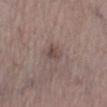Impression:
Imaged during a routine full-body skin examination; the lesion was not biopsied and no histopathology is available.
Background:
The lesion-visualizer software estimated a lesion color around L≈47 a*≈15 b*≈19 in CIELAB and roughly 7 lightness units darker than nearby skin. And it measured border irregularity of about 2 on a 0–10 scale, a within-lesion color-variation index near 4/10, and a peripheral color-asymmetry measure near 1.5. And it measured a lesion-detection confidence of about 100/100. The tile uses white-light illumination. A female patient, in their mid- to late 60s. A 15 mm close-up extracted from a 3D total-body photography capture. About 2.5 mm across. From the right lower leg.